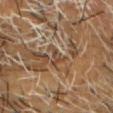{
  "biopsy_status": "not biopsied; imaged during a skin examination",
  "image": {
    "source": "total-body photography crop",
    "field_of_view_mm": 15
  },
  "site": "chest",
  "patient": {
    "sex": "male",
    "age_approx": 60
  }
}